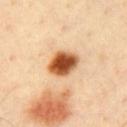This lesion was catalogued during total-body skin photography and was not selected for biopsy. A male patient about 40 years old. A 15 mm close-up tile from a total-body photography series done for melanoma screening. The lesion is on the chest.